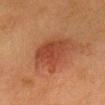<lesion>
<biopsy_status>not biopsied; imaged during a skin examination</biopsy_status>
<lesion_size>
  <long_diameter_mm_approx>5.5</long_diameter_mm_approx>
</lesion_size>
<image>
  <source>total-body photography crop</source>
  <field_of_view_mm>15</field_of_view_mm>
</image>
<site>head or neck</site>
<patient>
  <sex>female</sex>
  <age_approx>50</age_approx>
</patient>
<lighting>cross-polarized</lighting>
</lesion>